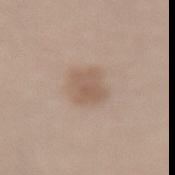The lesion was tiled from a total-body skin photograph and was not biopsied. Approximately 4 mm at its widest. A region of skin cropped from a whole-body photographic capture, roughly 15 mm wide. The lesion is on the lower back. Automated image analysis of the tile measured an area of roughly 9.5 mm², a shape eccentricity near 0.65, and two-axis asymmetry of about 0.2. The software also gave an automated nevus-likeness rating near 35 out of 100 and a detector confidence of about 100 out of 100 that the crop contains a lesion. A female subject aged around 25. This is a white-light tile.